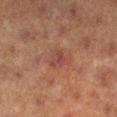Q: Was this lesion biopsied?
A: imaged on a skin check; not biopsied
Q: What is the lesion's diameter?
A: ~3.5 mm (longest diameter)
Q: Lesion location?
A: the left lower leg
Q: Automated lesion metrics?
A: an area of roughly 5 mm², a shape eccentricity near 0.65, and a symmetry-axis asymmetry near 0.3; a border-irregularity rating of about 3/10, a color-variation rating of about 3/10, and a peripheral color-asymmetry measure near 1; an automated nevus-likeness rating near 0 out of 100
Q: How was this image acquired?
A: ~15 mm crop, total-body skin-cancer survey
Q: What are the patient's age and sex?
A: female, aged 38–42
Q: What lighting was used for the tile?
A: cross-polarized illumination